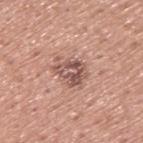The lesion was tiled from a total-body skin photograph and was not biopsied. The tile uses white-light illumination. A region of skin cropped from a whole-body photographic capture, roughly 15 mm wide. About 4 mm across. The lesion is on the upper back. The patient is a male in their mid- to late 40s.